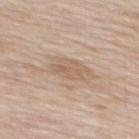Part of a total-body skin-imaging series; this lesion was reviewed on a skin check and was not flagged for biopsy. A male subject, in their 60s. Cropped from a whole-body photographic skin survey; the tile spans about 15 mm. Imaged with white-light lighting. An algorithmic analysis of the crop reported a footprint of about 8 mm², a shape eccentricity near 0.75, and two-axis asymmetry of about 0.2. It also reported a mean CIELAB color near L≈61 a*≈16 b*≈28 and a lesion-to-skin contrast of about 5 (normalized; higher = more distinct). And it measured a nevus-likeness score of about 0/100 and lesion-presence confidence of about 95/100. On the mid back. Longest diameter approximately 4 mm.